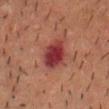workup: catalogued during a skin exam; not biopsied
automated metrics: a mean CIELAB color near L≈31 a*≈27 b*≈21, a lesion–skin lightness drop of about 11, and a normalized border contrast of about 11; a border-irregularity rating of about 2/10, a color-variation rating of about 4.5/10, and radial color variation of about 1; a classifier nevus-likeness of about 0/100 and lesion-presence confidence of about 100/100
image: ~15 mm tile from a whole-body skin photo
tile lighting: cross-polarized illumination
size: ~4 mm (longest diameter)
site: the head or neck
subject: male, about 45 years old